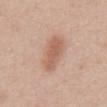  biopsy_status: not biopsied; imaged during a skin examination
  patient:
    sex: male
    age_approx: 50
  image:
    source: total-body photography crop
    field_of_view_mm: 15
  site: abdomen
  lesion_size:
    long_diameter_mm_approx: 5.0
  lighting: white-light